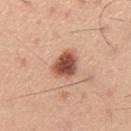Clinical impression:
Part of a total-body skin-imaging series; this lesion was reviewed on a skin check and was not flagged for biopsy.
Background:
Captured under white-light illumination. A male subject, in their mid- to late 40s. The lesion is located on the mid back. Automated image analysis of the tile measured a lesion area of about 8 mm² and an eccentricity of roughly 0.75. The software also gave an average lesion color of about L≈53 a*≈25 b*≈31 (CIELAB) and about 18 CIELAB-L* units darker than the surrounding skin. It also reported a border-irregularity index near 2/10. The software also gave a nevus-likeness score of about 100/100 and lesion-presence confidence of about 100/100. A lesion tile, about 15 mm wide, cut from a 3D total-body photograph.